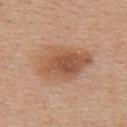follow-up: total-body-photography surveillance lesion; no biopsy
imaging modality: ~15 mm crop, total-body skin-cancer survey
location: the upper back
subject: female, roughly 40 years of age